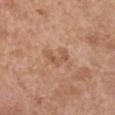Clinical summary:
The patient is a female roughly 65 years of age. From the arm. Imaged with white-light lighting. This image is a 15 mm lesion crop taken from a total-body photograph. Automated tile analysis of the lesion measured a footprint of about 4 mm², an outline eccentricity of about 0.8 (0 = round, 1 = elongated), and a symmetry-axis asymmetry near 0.6. About 3 mm across.